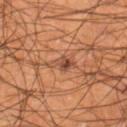Captured during whole-body skin photography for melanoma surveillance; the lesion was not biopsied.
A male patient, roughly 55 years of age.
A 15 mm close-up tile from a total-body photography series done for melanoma screening.
The lesion is on the leg.